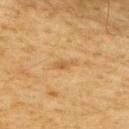Clinical impression:
The lesion was photographed on a routine skin check and not biopsied; there is no pathology result.
Image and clinical context:
This image is a 15 mm lesion crop taken from a total-body photograph. About 3 mm across. This is a cross-polarized tile. The subject is a male approximately 60 years of age. Located on the right upper arm.